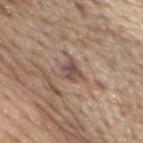biopsy status = imaged on a skin check; not biopsied | tile lighting = white-light illumination | body site = the chest | lesion diameter = about 2.5 mm | subject = male, aged 68 to 72 | image source = 15 mm crop, total-body photography.